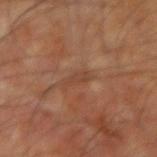No biopsy was performed on this lesion — it was imaged during a full skin examination and was not determined to be concerning. The lesion is located on the right forearm. A lesion tile, about 15 mm wide, cut from a 3D total-body photograph. A male patient, aged approximately 60.